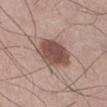Impression: The lesion was photographed on a routine skin check and not biopsied; there is no pathology result. Acquisition and patient details: Imaged with white-light lighting. From the right lower leg. Automated tile analysis of the lesion measured a footprint of about 15 mm² and an eccentricity of roughly 0.6. And it measured an average lesion color of about L≈50 a*≈19 b*≈22 (CIELAB), a lesion–skin lightness drop of about 15, and a lesion-to-skin contrast of about 10 (normalized; higher = more distinct). And it measured border irregularity of about 2 on a 0–10 scale. A male patient, roughly 35 years of age. This image is a 15 mm lesion crop taken from a total-body photograph. Longest diameter approximately 5 mm.